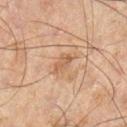biopsy_status: not biopsied; imaged during a skin examination
site: right lower leg
image:
  source: total-body photography crop
  field_of_view_mm: 15
lighting: cross-polarized
lesion_size:
  long_diameter_mm_approx: 2.5
patient:
  sex: male
  age_approx: 45
automated_metrics:
  nevus_likeness_0_100: 5
  lesion_detection_confidence_0_100: 100